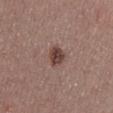workup = imaged on a skin check; not biopsied
subject = male, aged around 40
imaging modality = ~15 mm tile from a whole-body skin photo
location = the chest
lesion diameter = ≈3 mm
lighting = white-light